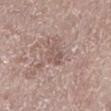follow-up — total-body-photography surveillance lesion; no biopsy
subject — male, aged 63–67
diameter — ~2.5 mm (longest diameter)
tile lighting — white-light
acquisition — total-body-photography crop, ~15 mm field of view
anatomic site — the left lower leg
automated lesion analysis — two-axis asymmetry of about 0.35; a mean CIELAB color near L≈54 a*≈17 b*≈22 and roughly 7 lightness units darker than nearby skin; a nevus-likeness score of about 0/100 and a lesion-detection confidence of about 55/100